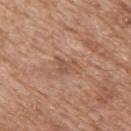{
  "biopsy_status": "not biopsied; imaged during a skin examination",
  "image": {
    "source": "total-body photography crop",
    "field_of_view_mm": 15
  },
  "automated_metrics": {
    "area_mm2_approx": 3.5,
    "shape_asymmetry": 0.45,
    "cielab_L": 52,
    "cielab_a": 21,
    "cielab_b": 30,
    "vs_skin_darker_L": 8.0,
    "vs_skin_contrast_norm": 5.5
  },
  "lesion_size": {
    "long_diameter_mm_approx": 2.5
  },
  "lighting": "white-light",
  "site": "upper back",
  "patient": {
    "sex": "male",
    "age_approx": 60
  }
}